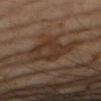workup: imaged on a skin check; not biopsied
automated metrics: a border-irregularity index near 4/10, a color-variation rating of about 3.5/10, and a peripheral color-asymmetry measure near 1; a classifier nevus-likeness of about 0/100 and lesion-presence confidence of about 60/100
illumination: cross-polarized
subject: female, about 60 years old
size: ≈6 mm
location: the right forearm
image source: ~15 mm crop, total-body skin-cancer survey